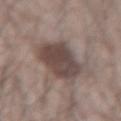Case summary:
– biopsy status: catalogued during a skin exam; not biopsied
– subject: male, roughly 25 years of age
– image: ~15 mm tile from a whole-body skin photo
– location: the left forearm
– tile lighting: white-light
– automated lesion analysis: an area of roughly 21 mm², an eccentricity of roughly 0.6, and a shape-asymmetry score of about 0.25 (0 = symmetric); an average lesion color of about L≈45 a*≈14 b*≈19 (CIELAB) and about 12 CIELAB-L* units darker than the surrounding skin; a border-irregularity index near 2.5/10, internal color variation of about 4 on a 0–10 scale, and peripheral color asymmetry of about 1.5
– lesion size: ≈6 mm